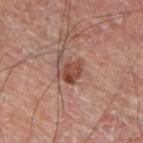follow-up: imaged on a skin check; not biopsied | subject: male, aged around 60 | image source: 15 mm crop, total-body photography | automated metrics: a border-irregularity index near 3/10, a within-lesion color-variation index near 4.5/10, and peripheral color asymmetry of about 2 | anatomic site: the right forearm | lighting: cross-polarized | lesion diameter: about 3 mm.